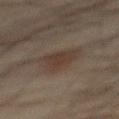workup — imaged on a skin check; not biopsied | subject — male, in their mid- to late 40s | body site — the abdomen | tile lighting — cross-polarized | TBP lesion metrics — a mean CIELAB color near L≈28 a*≈11 b*≈18, a lesion–skin lightness drop of about 5, and a lesion-to-skin contrast of about 6.5 (normalized; higher = more distinct); a border-irregularity index near 3.5/10 and internal color variation of about 2 on a 0–10 scale | image — ~15 mm crop, total-body skin-cancer survey | size — ~3 mm (longest diameter).